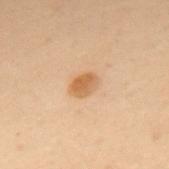Clinical impression: Imaged during a routine full-body skin examination; the lesion was not biopsied and no histopathology is available. Acquisition and patient details: The tile uses cross-polarized illumination. Located on the upper back. A roughly 15 mm field-of-view crop from a total-body skin photograph. Approximately 3 mm at its widest. A female patient aged around 50.